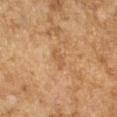Q: Was a biopsy performed?
A: total-body-photography surveillance lesion; no biopsy
Q: Automated lesion metrics?
A: a footprint of about 3 mm² and an outline eccentricity of about 0.9 (0 = round, 1 = elongated); about 6 CIELAB-L* units darker than the surrounding skin and a normalized lesion–skin contrast near 5; border irregularity of about 4 on a 0–10 scale, a within-lesion color-variation index near 0/10, and a peripheral color-asymmetry measure near 0
Q: Who is the patient?
A: female, aged 58 to 62
Q: What is the lesion's diameter?
A: ≈3 mm
Q: How was this image acquired?
A: ~15 mm crop, total-body skin-cancer survey
Q: How was the tile lit?
A: cross-polarized illumination
Q: Where on the body is the lesion?
A: the arm